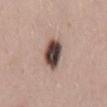{
  "biopsy_status": "not biopsied; imaged during a skin examination",
  "image": {
    "source": "total-body photography crop",
    "field_of_view_mm": 15
  },
  "lesion_size": {
    "long_diameter_mm_approx": 4.0
  },
  "patient": {
    "sex": "male",
    "age_approx": 25
  },
  "lighting": "white-light",
  "site": "mid back"
}